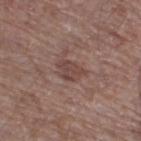follow-up = total-body-photography surveillance lesion; no biopsy
location = the right thigh
subject = male, aged approximately 70
acquisition = total-body-photography crop, ~15 mm field of view